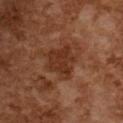Recorded during total-body skin imaging; not selected for excision or biopsy. On the upper back. The tile uses cross-polarized illumination. Longest diameter approximately 4 mm. A region of skin cropped from a whole-body photographic capture, roughly 15 mm wide. A female patient, aged 58 to 62.